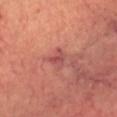Case summary:
* follow-up: imaged on a skin check; not biopsied
* image: 15 mm crop, total-body photography
* lighting: cross-polarized
* patient: male, aged around 65
* site: the head or neck
* lesion diameter: about 2.5 mm